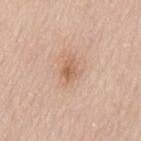The total-body-photography lesion software estimated an area of roughly 4.5 mm², an eccentricity of roughly 0.65, and a symmetry-axis asymmetry near 0.25. It also reported an average lesion color of about L≈61 a*≈20 b*≈32 (CIELAB) and a lesion–skin lightness drop of about 9. The software also gave radial color variation of about 1.5. A region of skin cropped from a whole-body photographic capture, roughly 15 mm wide. About 2.5 mm across. The lesion is on the mid back. A male subject aged 63–67. The tile uses white-light illumination.